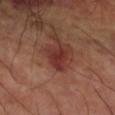| key | value |
|---|---|
| biopsy status | catalogued during a skin exam; not biopsied |
| location | the left upper arm |
| image | ~15 mm tile from a whole-body skin photo |
| subject | male, aged approximately 60 |
| tile lighting | cross-polarized |